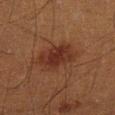Captured under cross-polarized illumination. A male patient approximately 40 years of age. Longest diameter approximately 4 mm. From the left lower leg. A 15 mm close-up tile from a total-body photography series done for melanoma screening. An algorithmic analysis of the crop reported a lesion area of about 12 mm² and an eccentricity of roughly 0.6. It also reported border irregularity of about 2.5 on a 0–10 scale, a within-lesion color-variation index near 3/10, and a peripheral color-asymmetry measure near 1.